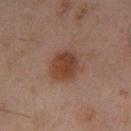<record>
  <biopsy_status>not biopsied; imaged during a skin examination</biopsy_status>
  <patient>
    <sex>male</sex>
    <age_approx>45</age_approx>
  </patient>
  <site>left lower leg</site>
  <image>
    <source>total-body photography crop</source>
    <field_of_view_mm>15</field_of_view_mm>
  </image>
  <lesion_size>
    <long_diameter_mm_approx>4.0</long_diameter_mm_approx>
  </lesion_size>
</record>